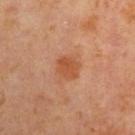biopsy_status: not biopsied; imaged during a skin examination
patient:
  sex: male
  age_approx: 60
automated_metrics:
  border_irregularity_0_10: 2.0
  color_variation_0_10: 2.0
  peripheral_color_asymmetry: 0.5
  nevus_likeness_0_100: 40
  lesion_detection_confidence_0_100: 100
site: left upper arm
image:
  source: total-body photography crop
  field_of_view_mm: 15
lighting: cross-polarized